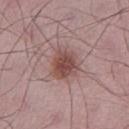Clinical impression:
Captured during whole-body skin photography for melanoma surveillance; the lesion was not biopsied.
Image and clinical context:
The total-body-photography lesion software estimated a footprint of about 9.5 mm² and a shape eccentricity near 0.5. The software also gave a classifier nevus-likeness of about 90/100 and a detector confidence of about 100 out of 100 that the crop contains a lesion. Cropped from a total-body skin-imaging series; the visible field is about 15 mm. Measured at roughly 3.5 mm in maximum diameter. A male subject, aged 48–52. Located on the left lower leg.